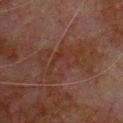The lesion was photographed on a routine skin check and not biopsied; there is no pathology result.
The lesion's longest dimension is about 8 mm.
The subject is a male in their 80s.
A roughly 15 mm field-of-view crop from a total-body skin photograph.
The lesion is on the front of the torso.